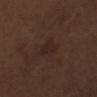follow-up — no biopsy performed (imaged during a skin exam) | image-analysis metrics — an area of roughly 5 mm² and two-axis asymmetry of about 0.35; about 4 CIELAB-L* units darker than the surrounding skin and a normalized border contrast of about 5.5; a classifier nevus-likeness of about 5/100 and a detector confidence of about 100 out of 100 that the crop contains a lesion | tile lighting — white-light illumination | site — the right upper arm | patient — male, aged 68 to 72 | diameter — ≈3.5 mm | acquisition — ~15 mm crop, total-body skin-cancer survey.